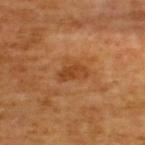A male patient in their mid- to late 60s. On the back. The recorded lesion diameter is about 3.5 mm. This is a cross-polarized tile. A region of skin cropped from a whole-body photographic capture, roughly 15 mm wide. The total-body-photography lesion software estimated an eccentricity of roughly 0.8 and a shape-asymmetry score of about 0.35 (0 = symmetric). It also reported an average lesion color of about L≈43 a*≈25 b*≈39 (CIELAB), a lesion–skin lightness drop of about 8, and a lesion-to-skin contrast of about 7 (normalized; higher = more distinct). And it measured a nevus-likeness score of about 15/100.